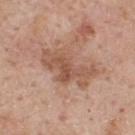Clinical impression: Recorded during total-body skin imaging; not selected for excision or biopsy. Background: This image is a 15 mm lesion crop taken from a total-body photograph. Captured under white-light illumination. On the upper back. A male patient, roughly 60 years of age.